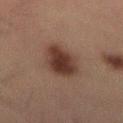Background:
On the back. A region of skin cropped from a whole-body photographic capture, roughly 15 mm wide. The patient is a male aged approximately 50.